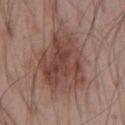biopsy status: no biopsy performed (imaged during a skin exam)
lighting: white-light illumination
image source: ~15 mm tile from a whole-body skin photo
lesion size: about 7 mm
patient: male, aged 53 to 57
TBP lesion metrics: roughly 10 lightness units darker than nearby skin and a lesion-to-skin contrast of about 7.5 (normalized; higher = more distinct); a classifier nevus-likeness of about 45/100 and lesion-presence confidence of about 100/100
location: the chest